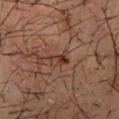Assessment: No biopsy was performed on this lesion — it was imaged during a full skin examination and was not determined to be concerning.